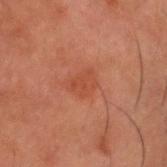A male subject approximately 50 years of age. Longest diameter approximately 3 mm. From the head or neck. A 15 mm close-up tile from a total-body photography series done for melanoma screening.